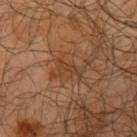Assessment: The lesion was photographed on a routine skin check and not biopsied; there is no pathology result. Image and clinical context: A 15 mm close-up tile from a total-body photography series done for melanoma screening. Captured under cross-polarized illumination. Automated tile analysis of the lesion measured a mean CIELAB color near L≈38 a*≈20 b*≈30 and a normalized lesion–skin contrast near 5.5. It also reported a nevus-likeness score of about 0/100 and a lesion-detection confidence of about 60/100. On the left upper arm. The patient is a male in their mid- to late 60s.